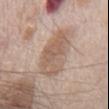Recorded during total-body skin imaging; not selected for excision or biopsy. The lesion's longest dimension is about 6.5 mm. A lesion tile, about 15 mm wide, cut from a 3D total-body photograph. This is a white-light tile. Located on the chest. A male patient, aged 68–72.